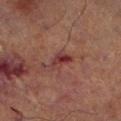biopsy_status: not biopsied; imaged during a skin examination
lighting: cross-polarized
image:
  source: total-body photography crop
  field_of_view_mm: 15
site: left lower leg
patient:
  sex: male
  age_approx: 70